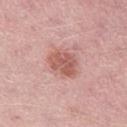Clinical impression:
This lesion was catalogued during total-body skin photography and was not selected for biopsy.
Image and clinical context:
On the left thigh. A male subject, aged 48 to 52. A close-up tile cropped from a whole-body skin photograph, about 15 mm across.